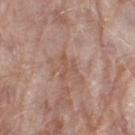{"biopsy_status": "not biopsied; imaged during a skin examination", "site": "right thigh", "image": {"source": "total-body photography crop", "field_of_view_mm": 15}, "lesion_size": {"long_diameter_mm_approx": 3.0}, "patient": {"sex": "female", "age_approx": 70}}